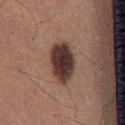Recorded during total-body skin imaging; not selected for excision or biopsy. The lesion's longest dimension is about 5 mm. Automated image analysis of the tile measured a lesion color around L≈35 a*≈19 b*≈21 in CIELAB and a normalized border contrast of about 15. The analysis additionally found a border-irregularity rating of about 1.5/10, a within-lesion color-variation index near 5/10, and radial color variation of about 1. A roughly 15 mm field-of-view crop from a total-body skin photograph. The tile uses white-light illumination. A male patient approximately 40 years of age. On the mid back.